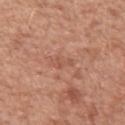follow-up=imaged on a skin check; not biopsied
acquisition=total-body-photography crop, ~15 mm field of view
body site=the left upper arm
subject=male, aged approximately 50